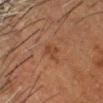biopsy_status: not biopsied; imaged during a skin examination
patient:
  sex: male
  age_approx: 50
site: head or neck
automated_metrics:
  eccentricity: 0.75
  shape_asymmetry: 0.35
  cielab_L: 33
  cielab_a: 19
  cielab_b: 27
  vs_skin_darker_L: 5.0
  border_irregularity_0_10: 3.5
  color_variation_0_10: 1.5
  lesion_detection_confidence_0_100: 100
image:
  source: total-body photography crop
  field_of_view_mm: 15